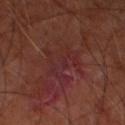No biopsy was performed on this lesion — it was imaged during a full skin examination and was not determined to be concerning.
From the left thigh.
The patient is a male in their mid- to late 60s.
Cropped from a total-body skin-imaging series; the visible field is about 15 mm.
Imaged with cross-polarized lighting.
The recorded lesion diameter is about 5.5 mm.